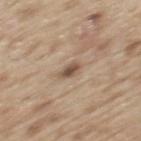<record>
  <biopsy_status>not biopsied; imaged during a skin examination</biopsy_status>
</record>